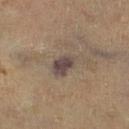follow-up=no biopsy performed (imaged during a skin exam)
size=about 3.5 mm
tile lighting=cross-polarized illumination
acquisition=~15 mm crop, total-body skin-cancer survey
subject=roughly 60 years of age
anatomic site=the right lower leg